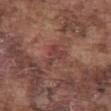| feature | finding |
|---|---|
| notes | catalogued during a skin exam; not biopsied |
| lesion size | about 3.5 mm |
| illumination | white-light |
| site | the abdomen |
| TBP lesion metrics | a shape eccentricity near 0.7 and a shape-asymmetry score of about 0.65 (0 = symmetric); an average lesion color of about L≈41 a*≈23 b*≈23 (CIELAB), a lesion–skin lightness drop of about 6, and a normalized lesion–skin contrast near 5.5; a border-irregularity index near 7/10, internal color variation of about 1.5 on a 0–10 scale, and radial color variation of about 0.5; a nevus-likeness score of about 0/100 and a lesion-detection confidence of about 80/100 |
| subject | male, about 75 years old |
| image | ~15 mm crop, total-body skin-cancer survey |